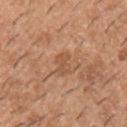Q: Is there a histopathology result?
A: no biopsy performed (imaged during a skin exam)
Q: How large is the lesion?
A: ≈2.5 mm
Q: Patient demographics?
A: male, about 40 years old
Q: What is the anatomic site?
A: the chest
Q: What lighting was used for the tile?
A: white-light
Q: What kind of image is this?
A: 15 mm crop, total-body photography